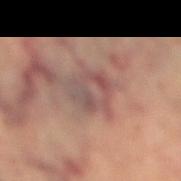Imaged during a routine full-body skin examination; the lesion was not biopsied and no histopathology is available.
From the left leg.
The subject is a female aged 63 to 67.
A roughly 15 mm field-of-view crop from a total-body skin photograph.